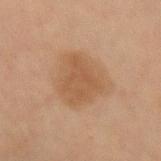Assessment:
Imaged during a routine full-body skin examination; the lesion was not biopsied and no histopathology is available.
Clinical summary:
A female patient in their 40s. The tile uses cross-polarized illumination. The lesion-visualizer software estimated an area of roughly 20 mm² and a shape-asymmetry score of about 0.25 (0 = symmetric). And it measured a mean CIELAB color near L≈47 a*≈17 b*≈30, about 7 CIELAB-L* units darker than the surrounding skin, and a normalized lesion–skin contrast near 6. And it measured a classifier nevus-likeness of about 55/100. Measured at roughly 5.5 mm in maximum diameter. From the left forearm. Cropped from a total-body skin-imaging series; the visible field is about 15 mm.